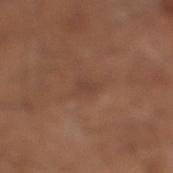Assessment: This lesion was catalogued during total-body skin photography and was not selected for biopsy. Context: Cropped from a total-body skin-imaging series; the visible field is about 15 mm. Captured under white-light illumination. Located on the right lower leg. The recorded lesion diameter is about 2.5 mm. A male patient in their mid- to late 60s.